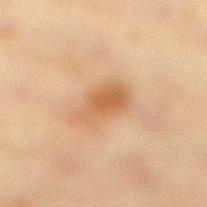The lesion was tiled from a total-body skin photograph and was not biopsied. The lesion is on the right lower leg. About 5 mm across. A female patient roughly 40 years of age. A 15 mm close-up tile from a total-body photography series done for melanoma screening. This is a cross-polarized tile.